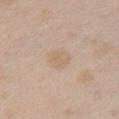workup=total-body-photography surveillance lesion; no biopsy
location=the chest
subject=female, aged 23 to 27
size=about 2.5 mm
acquisition=15 mm crop, total-body photography
image-analysis metrics=an area of roughly 3.5 mm², a shape eccentricity near 0.75, and a symmetry-axis asymmetry near 0.35; a lesion color around L≈64 a*≈15 b*≈31 in CIELAB, about 5 CIELAB-L* units darker than the surrounding skin, and a normalized lesion–skin contrast near 5; a border-irregularity index near 3.5/10 and a color-variation rating of about 1/10
illumination=white-light